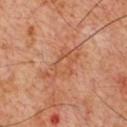{
  "biopsy_status": "not biopsied; imaged during a skin examination",
  "patient": {
    "sex": "male",
    "age_approx": 60
  },
  "lesion_size": {
    "long_diameter_mm_approx": 6.0
  },
  "lighting": "cross-polarized",
  "site": "chest",
  "image": {
    "source": "total-body photography crop",
    "field_of_view_mm": 15
  }
}